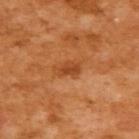Assessment:
No biopsy was performed on this lesion — it was imaged during a full skin examination and was not determined to be concerning.
Image and clinical context:
This is a cross-polarized tile. A female patient, in their mid-50s. Located on the back. Measured at roughly 2.5 mm in maximum diameter. A 15 mm close-up tile from a total-body photography series done for melanoma screening.